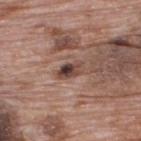Q: Was a biopsy performed?
A: no biopsy performed (imaged during a skin exam)
Q: Illumination type?
A: white-light illumination
Q: Who is the patient?
A: male, approximately 70 years of age
Q: Where on the body is the lesion?
A: the back
Q: How large is the lesion?
A: about 3 mm
Q: What did automated image analysis measure?
A: a footprint of about 4.5 mm² and a shape-asymmetry score of about 0.25 (0 = symmetric); a lesion color around L≈43 a*≈19 b*≈22 in CIELAB and about 13 CIELAB-L* units darker than the surrounding skin
Q: How was this image acquired?
A: ~15 mm crop, total-body skin-cancer survey